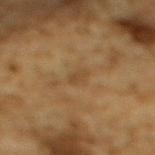* biopsy status · imaged on a skin check; not biopsied
* body site · the upper back
* patient · male, aged approximately 85 or older
* image source · total-body-photography crop, ~15 mm field of view